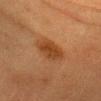The lesion was tiled from a total-body skin photograph and was not biopsied. A roughly 15 mm field-of-view crop from a total-body skin photograph. A female patient, in their mid-50s. Located on the head or neck.